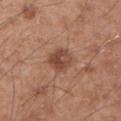The lesion was tiled from a total-body skin photograph and was not biopsied.
A male subject, aged approximately 55.
The recorded lesion diameter is about 3 mm.
This image is a 15 mm lesion crop taken from a total-body photograph.
The lesion is located on the arm.
Automated image analysis of the tile measured a shape-asymmetry score of about 0.15 (0 = symmetric). The software also gave a lesion color around L≈46 a*≈22 b*≈28 in CIELAB and a normalized border contrast of about 8.
Imaged with white-light lighting.